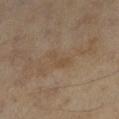Clinical impression: Imaged during a routine full-body skin examination; the lesion was not biopsied and no histopathology is available. Context: Captured under cross-polarized illumination. This image is a 15 mm lesion crop taken from a total-body photograph. The total-body-photography lesion software estimated a lesion color around L≈42 a*≈12 b*≈27 in CIELAB, about 4 CIELAB-L* units darker than the surrounding skin, and a normalized lesion–skin contrast near 4.5. The software also gave a classifier nevus-likeness of about 0/100. The lesion is on the leg. The patient is a female aged 58 to 62. Approximately 3 mm at its widest.